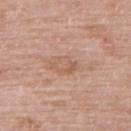Imaged during a routine full-body skin examination; the lesion was not biopsied and no histopathology is available. A female patient in their mid-80s or older. The lesion is located on the back. A roughly 15 mm field-of-view crop from a total-body skin photograph.